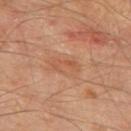| feature | finding |
|---|---|
| follow-up | catalogued during a skin exam; not biopsied |
| location | the mid back |
| illumination | cross-polarized |
| image | ~15 mm crop, total-body skin-cancer survey |
| subject | male, in their mid- to late 60s |